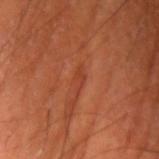Q: Was a biopsy performed?
A: total-body-photography surveillance lesion; no biopsy
Q: Lesion location?
A: the left upper arm
Q: Patient demographics?
A: male, aged around 60
Q: How was this image acquired?
A: total-body-photography crop, ~15 mm field of view
Q: How was the tile lit?
A: cross-polarized illumination
Q: What did automated image analysis measure?
A: a shape eccentricity near 0.95; border irregularity of about 4 on a 0–10 scale, a within-lesion color-variation index near 0/10, and peripheral color asymmetry of about 0; a detector confidence of about 65 out of 100 that the crop contains a lesion
Q: How large is the lesion?
A: about 3 mm